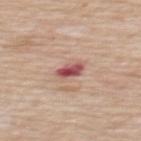Impression:
Recorded during total-body skin imaging; not selected for excision or biopsy.
Context:
A roughly 15 mm field-of-view crop from a total-body skin photograph. Automated tile analysis of the lesion measured border irregularity of about 2 on a 0–10 scale, a within-lesion color-variation index near 6/10, and a peripheral color-asymmetry measure near 2. Approximately 2.5 mm at its widest. Located on the mid back. The patient is a male approximately 65 years of age.